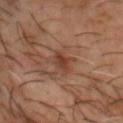biopsy_status: not biopsied; imaged during a skin examination
site: head or neck
lesion_size:
  long_diameter_mm_approx: 2.5
patient:
  sex: male
  age_approx: 50
image:
  source: total-body photography crop
  field_of_view_mm: 15
lighting: cross-polarized